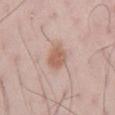Part of a total-body skin-imaging series; this lesion was reviewed on a skin check and was not flagged for biopsy. A 15 mm crop from a total-body photograph taken for skin-cancer surveillance. The lesion-visualizer software estimated a border-irregularity rating of about 2/10 and a within-lesion color-variation index near 1.5/10. And it measured a nevus-likeness score of about 80/100 and a lesion-detection confidence of about 100/100. The lesion's longest dimension is about 3 mm. Located on the abdomen. A male patient, aged 43 to 47.